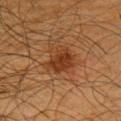workup = no biopsy performed (imaged during a skin exam); automated metrics = a mean CIELAB color near L≈30 a*≈20 b*≈30 and about 8 CIELAB-L* units darker than the surrounding skin; tile lighting = cross-polarized; image source = total-body-photography crop, ~15 mm field of view; diameter = about 4 mm; subject = male, roughly 65 years of age; location = the left upper arm.